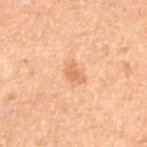• follow-up: imaged on a skin check; not biopsied
• lesion diameter: ≈3 mm
• location: the right thigh
• lighting: cross-polarized illumination
• subject: male, aged 68–72
• imaging modality: 15 mm crop, total-body photography
• image-analysis metrics: a nevus-likeness score of about 20/100 and lesion-presence confidence of about 100/100